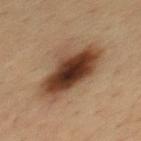| key | value |
|---|---|
| follow-up | total-body-photography surveillance lesion; no biopsy |
| size | ≈7 mm |
| image | 15 mm crop, total-body photography |
| patient | male, about 35 years old |
| lighting | cross-polarized |
| location | the mid back |